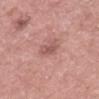notes — no biopsy performed (imaged during a skin exam) | patient — male, about 60 years old | diameter — ~2.5 mm (longest diameter) | TBP lesion metrics — a footprint of about 4 mm² and two-axis asymmetry of about 0.35; an automated nevus-likeness rating near 0 out of 100 and lesion-presence confidence of about 100/100 | anatomic site — the abdomen | image — 15 mm crop, total-body photography | lighting — white-light illumination.